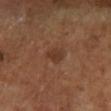Acquisition and patient details:
A female patient, aged around 65. The lesion's longest dimension is about 2.5 mm. Cropped from a total-body skin-imaging series; the visible field is about 15 mm. Captured under cross-polarized illumination. From the left lower leg.